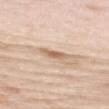Q: What is the imaging modality?
A: ~15 mm crop, total-body skin-cancer survey
Q: What is the lesion's diameter?
A: about 3 mm
Q: Where on the body is the lesion?
A: the upper back
Q: What lighting was used for the tile?
A: white-light illumination
Q: Patient demographics?
A: female, aged approximately 50